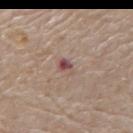This lesion was catalogued during total-body skin photography and was not selected for biopsy.
The lesion is on the mid back.
The recorded lesion diameter is about 2.5 mm.
Automated image analysis of the tile measured an eccentricity of roughly 0.8 and a symmetry-axis asymmetry near 0.25. It also reported a mean CIELAB color near L≈50 a*≈20 b*≈21, about 10 CIELAB-L* units darker than the surrounding skin, and a lesion-to-skin contrast of about 7.5 (normalized; higher = more distinct). The analysis additionally found border irregularity of about 2.5 on a 0–10 scale and radial color variation of about 1.5.
A lesion tile, about 15 mm wide, cut from a 3D total-body photograph.
Imaged with white-light lighting.
The subject is a male in their 80s.